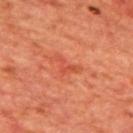No biopsy was performed on this lesion — it was imaged during a full skin examination and was not determined to be concerning.
A 15 mm crop from a total-body photograph taken for skin-cancer surveillance.
The lesion is located on the mid back.
A male subject aged 63–67.
This is a cross-polarized tile.